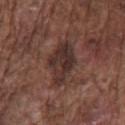The lesion was tiled from a total-body skin photograph and was not biopsied.
Approximately 6 mm at its widest.
The lesion-visualizer software estimated a mean CIELAB color near L≈32 a*≈17 b*≈20 and a normalized border contrast of about 9. The analysis additionally found an automated nevus-likeness rating near 0 out of 100 and a detector confidence of about 55 out of 100 that the crop contains a lesion.
The lesion is located on the chest.
A close-up tile cropped from a whole-body skin photograph, about 15 mm across.
A male patient, approximately 75 years of age.
The tile uses white-light illumination.